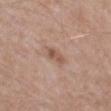Notes:
- biopsy status · no biopsy performed (imaged during a skin exam)
- anatomic site · the left thigh
- acquisition · 15 mm crop, total-body photography
- image-analysis metrics · a mean CIELAB color near L≈53 a*≈19 b*≈27, about 10 CIELAB-L* units darker than the surrounding skin, and a normalized border contrast of about 7; a nevus-likeness score of about 5/100 and a lesion-detection confidence of about 100/100
- patient · male, aged approximately 65
- lesion diameter · about 3 mm
- lighting · white-light